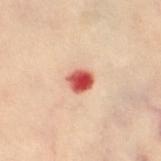{
  "biopsy_status": "not biopsied; imaged during a skin examination"
}